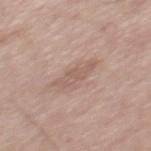<lesion>
<biopsy_status>not biopsied; imaged during a skin examination</biopsy_status>
<lesion_size>
  <long_diameter_mm_approx>4.0</long_diameter_mm_approx>
</lesion_size>
<image>
  <source>total-body photography crop</source>
  <field_of_view_mm>15</field_of_view_mm>
</image>
<automated_metrics>
  <cielab_L>57</cielab_L>
  <cielab_a>18</cielab_a>
  <cielab_b>25</cielab_b>
  <vs_skin_darker_L>7.0</vs_skin_darker_L>
  <vs_skin_contrast_norm>5.0</vs_skin_contrast_norm>
  <border_irregularity_0_10>4.5</border_irregularity_0_10>
  <color_variation_0_10>1.0</color_variation_0_10>
  <peripheral_color_asymmetry>0.5</peripheral_color_asymmetry>
</automated_metrics>
<lighting>white-light</lighting>
<site>mid back</site>
<patient>
  <sex>male</sex>
  <age_approx>55</age_approx>
</patient>
</lesion>